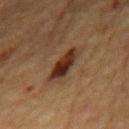Q: Is there a histopathology result?
A: imaged on a skin check; not biopsied
Q: What is the lesion's diameter?
A: ~5.5 mm (longest diameter)
Q: What lighting was used for the tile?
A: cross-polarized illumination
Q: What are the patient's age and sex?
A: male, in their mid-80s or older
Q: Automated lesion metrics?
A: an average lesion color of about L≈28 a*≈16 b*≈26 (CIELAB), roughly 11 lightness units darker than nearby skin, and a lesion-to-skin contrast of about 11 (normalized; higher = more distinct); a detector confidence of about 100 out of 100 that the crop contains a lesion
Q: Lesion location?
A: the chest
Q: What is the imaging modality?
A: ~15 mm tile from a whole-body skin photo